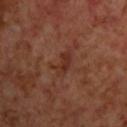Impression: Imaged during a routine full-body skin examination; the lesion was not biopsied and no histopathology is available. Acquisition and patient details: Automated image analysis of the tile measured a footprint of about 5 mm², an eccentricity of roughly 0.85, and a shape-asymmetry score of about 0.45 (0 = symmetric). It also reported border irregularity of about 5.5 on a 0–10 scale, a color-variation rating of about 3/10, and radial color variation of about 1. The analysis additionally found a lesion-detection confidence of about 95/100. A male subject, in their 70s. From the upper back. About 3.5 mm across. A 15 mm close-up tile from a total-body photography series done for melanoma screening. Captured under cross-polarized illumination.